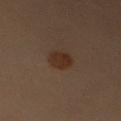The lesion was photographed on a routine skin check and not biopsied; there is no pathology result.
An algorithmic analysis of the crop reported an average lesion color of about L≈22 a*≈14 b*≈20 (CIELAB), a lesion–skin lightness drop of about 6, and a lesion-to-skin contrast of about 8.5 (normalized; higher = more distinct). The software also gave border irregularity of about 2 on a 0–10 scale and peripheral color asymmetry of about 0.5.
This is a cross-polarized tile.
The lesion is on the arm.
A 15 mm close-up extracted from a 3D total-body photography capture.
The subject is a female roughly 40 years of age.
Approximately 3.5 mm at its widest.